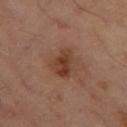follow-up = no biopsy performed (imaged during a skin exam) | diameter = ≈4 mm | anatomic site = the left thigh | illumination = cross-polarized | imaging modality = 15 mm crop, total-body photography.